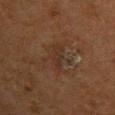notes: imaged on a skin check; not biopsied
acquisition: total-body-photography crop, ~15 mm field of view
automated lesion analysis: a lesion area of about 3.5 mm² and a symmetry-axis asymmetry near 0.3; an automated nevus-likeness rating near 0 out of 100 and a lesion-detection confidence of about 70/100
subject: female, about 50 years old
anatomic site: the chest
lighting: cross-polarized